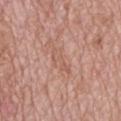biopsy status = catalogued during a skin exam; not biopsied
body site = the mid back
diameter = ~4 mm (longest diameter)
patient = male, in their 70s
image source = 15 mm crop, total-body photography